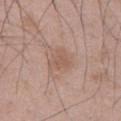lesion size: ≈3 mm
subject: male, in their 50s
acquisition: 15 mm crop, total-body photography
automated lesion analysis: a mean CIELAB color near L≈56 a*≈18 b*≈27 and a lesion–skin lightness drop of about 7; a nevus-likeness score of about 5/100 and a lesion-detection confidence of about 100/100
site: the abdomen
illumination: white-light illumination